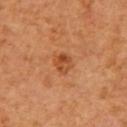  image:
    source: total-body photography crop
    field_of_view_mm: 15
  lesion_size:
    long_diameter_mm_approx: 2.5
  automated_metrics:
    eccentricity: 0.55
    shape_asymmetry: 0.25
    nevus_likeness_0_100: 65
    lesion_detection_confidence_0_100: 100
  patient:
    sex: male
    age_approx: 60
  lighting: cross-polarized
  site: right upper arm